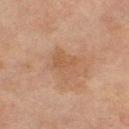follow-up: total-body-photography surveillance lesion; no biopsy | image source: total-body-photography crop, ~15 mm field of view | diameter: ≈4 mm | subject: female, approximately 70 years of age | body site: the right thigh | lighting: cross-polarized | automated lesion analysis: a footprint of about 7.5 mm² and two-axis asymmetry of about 0.35; a nevus-likeness score of about 0/100 and lesion-presence confidence of about 100/100.